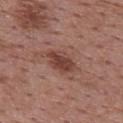{"biopsy_status": "not biopsied; imaged during a skin examination", "lesion_size": {"long_diameter_mm_approx": 4.0}, "site": "back", "patient": {"sex": "female", "age_approx": 50}, "image": {"source": "total-body photography crop", "field_of_view_mm": 15}, "lighting": "white-light"}